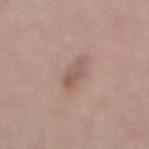* biopsy status: catalogued during a skin exam; not biopsied
* lighting: white-light
* imaging modality: total-body-photography crop, ~15 mm field of view
* site: the right lower leg
* subject: female, about 40 years old